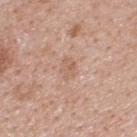Captured during whole-body skin photography for melanoma surveillance; the lesion was not biopsied.
A male patient about 40 years old.
Located on the back.
A roughly 15 mm field-of-view crop from a total-body skin photograph.